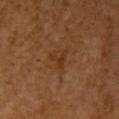Captured during whole-body skin photography for melanoma surveillance; the lesion was not biopsied. Automated image analysis of the tile measured internal color variation of about 0 on a 0–10 scale and a peripheral color-asymmetry measure near 0. And it measured an automated nevus-likeness rating near 0 out of 100 and a lesion-detection confidence of about 100/100. Cropped from a whole-body photographic skin survey; the tile spans about 15 mm. This is a cross-polarized tile. On the right upper arm. The recorded lesion diameter is about 2.5 mm. A female subject, approximately 55 years of age.